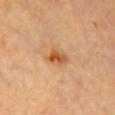Clinical impression:
Imaged during a routine full-body skin examination; the lesion was not biopsied and no histopathology is available.
Clinical summary:
A 15 mm crop from a total-body photograph taken for skin-cancer surveillance. Approximately 3 mm at its widest. From the chest. A female subject, aged approximately 60. Imaged with cross-polarized lighting. Automated tile analysis of the lesion measured about 11 CIELAB-L* units darker than the surrounding skin and a normalized border contrast of about 8. It also reported a border-irregularity index near 3/10 and radial color variation of about 2.5. The analysis additionally found a nevus-likeness score of about 90/100 and a lesion-detection confidence of about 100/100.